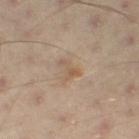{"lighting": "cross-polarized", "site": "right thigh", "image": {"source": "total-body photography crop", "field_of_view_mm": 15}, "patient": {"sex": "male", "age_approx": 55}}